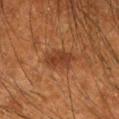follow-up — total-body-photography surveillance lesion; no biopsy | site — the right forearm | automated metrics — an area of roughly 7 mm² and two-axis asymmetry of about 0.2; a border-irregularity rating of about 2.5/10 and radial color variation of about 1; a classifier nevus-likeness of about 65/100 and a lesion-detection confidence of about 100/100 | tile lighting — cross-polarized | image — 15 mm crop, total-body photography | diameter — about 4 mm | subject — male, in their mid- to late 60s.